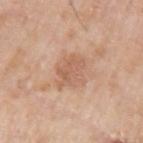| key | value |
|---|---|
| biopsy status | imaged on a skin check; not biopsied |
| lighting | white-light illumination |
| subject | male, approximately 70 years of age |
| acquisition | ~15 mm tile from a whole-body skin photo |
| site | the arm |
| lesion diameter | ≈4 mm |
| automated metrics | a mean CIELAB color near L≈60 a*≈21 b*≈31 and about 8 CIELAB-L* units darker than the surrounding skin; an automated nevus-likeness rating near 0 out of 100 |